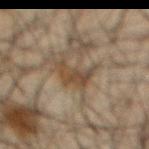Clinical impression:
Recorded during total-body skin imaging; not selected for excision or biopsy.
Clinical summary:
The lesion is located on the chest. The patient is a male roughly 65 years of age. A 15 mm crop from a total-body photograph taken for skin-cancer surveillance. The lesion's longest dimension is about 4 mm. This is a cross-polarized tile. Automated tile analysis of the lesion measured an area of roughly 7 mm², an outline eccentricity of about 0.55 (0 = round, 1 = elongated), and two-axis asymmetry of about 0.5. And it measured internal color variation of about 2.5 on a 0–10 scale and peripheral color asymmetry of about 1. The analysis additionally found a detector confidence of about 60 out of 100 that the crop contains a lesion.